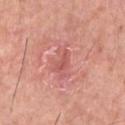Q: Is there a histopathology result?
A: no biopsy performed (imaged during a skin exam)
Q: How was this image acquired?
A: 15 mm crop, total-body photography
Q: Lesion location?
A: the chest
Q: Patient demographics?
A: male, approximately 45 years of age